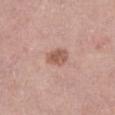Imaged during a routine full-body skin examination; the lesion was not biopsied and no histopathology is available. Automated image analysis of the tile measured an area of roughly 5 mm², a shape eccentricity near 0.75, and a symmetry-axis asymmetry near 0.2. The software also gave a lesion color around L≈56 a*≈21 b*≈28 in CIELAB, roughly 11 lightness units darker than nearby skin, and a normalized border contrast of about 7.5. It also reported a border-irregularity index near 2/10, a color-variation rating of about 2.5/10, and peripheral color asymmetry of about 1. The software also gave a detector confidence of about 100 out of 100 that the crop contains a lesion. A female patient in their 40s. Located on the right thigh. Approximately 3 mm at its widest. A 15 mm close-up tile from a total-body photography series done for melanoma screening.